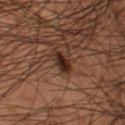Notes:
* biopsy status: imaged on a skin check; not biopsied
* location: the left thigh
* size: about 2.5 mm
* patient: male, aged 58–62
* acquisition: 15 mm crop, total-body photography
* automated lesion analysis: a lesion area of about 3.5 mm², an eccentricity of roughly 0.75, and a symmetry-axis asymmetry near 0.25
* lighting: cross-polarized